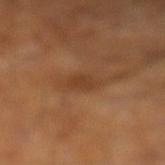biopsy status — imaged on a skin check; not biopsied
tile lighting — cross-polarized illumination
anatomic site — the left lower leg
image source — 15 mm crop, total-body photography
patient — male, aged around 60
lesion size — ≈3 mm